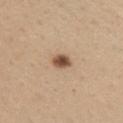– notes: total-body-photography surveillance lesion; no biopsy
– tile lighting: white-light illumination
– location: the front of the torso
– subject: female, aged 58 to 62
– lesion size: ≈2.5 mm
– image: 15 mm crop, total-body photography